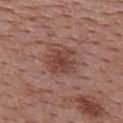location = the upper back
lighting = white-light
diameter = ~3.5 mm (longest diameter)
imaging modality = ~15 mm crop, total-body skin-cancer survey
subject = female, in their 50s
automated lesion analysis = an area of roughly 9.5 mm² and a symmetry-axis asymmetry near 0.2; a nevus-likeness score of about 40/100 and a lesion-detection confidence of about 100/100